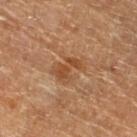Q: Was this lesion biopsied?
A: catalogued during a skin exam; not biopsied
Q: How was the tile lit?
A: cross-polarized illumination
Q: Who is the patient?
A: male, aged around 85 or older
Q: Automated lesion metrics?
A: a mean CIELAB color near L≈48 a*≈21 b*≈34, about 7 CIELAB-L* units darker than the surrounding skin, and a lesion-to-skin contrast of about 6 (normalized; higher = more distinct); an automated nevus-likeness rating near 0 out of 100 and a detector confidence of about 100 out of 100 that the crop contains a lesion
Q: What is the lesion's diameter?
A: about 4 mm
Q: What kind of image is this?
A: ~15 mm crop, total-body skin-cancer survey
Q: Where on the body is the lesion?
A: the left lower leg